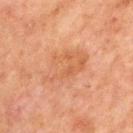notes: total-body-photography surveillance lesion; no biopsy | imaging modality: total-body-photography crop, ~15 mm field of view | diameter: ≈6 mm | subject: male, aged 63 to 67 | anatomic site: the chest | lighting: cross-polarized | TBP lesion metrics: a shape eccentricity near 0.85 and a symmetry-axis asymmetry near 0.25; internal color variation of about 3.5 on a 0–10 scale and peripheral color asymmetry of about 1; a classifier nevus-likeness of about 0/100.